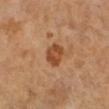The lesion was tiled from a total-body skin photograph and was not biopsied. A female patient, aged approximately 55. From the right lower leg. A 15 mm close-up tile from a total-body photography series done for melanoma screening.